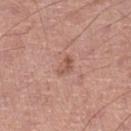No biopsy was performed on this lesion — it was imaged during a full skin examination and was not determined to be concerning. A male patient about 65 years old. This image is a 15 mm lesion crop taken from a total-body photograph. From the leg. The lesion's longest dimension is about 2.5 mm. This is a white-light tile.